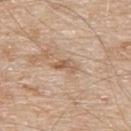Captured under white-light illumination. The lesion is located on the upper back. Cropped from a whole-body photographic skin survey; the tile spans about 15 mm. An algorithmic analysis of the crop reported an eccentricity of roughly 0.9. The analysis additionally found a border-irregularity index near 4.5/10, a within-lesion color-variation index near 1.5/10, and a peripheral color-asymmetry measure near 0.5. The patient is a male roughly 80 years of age. Longest diameter approximately 3 mm.